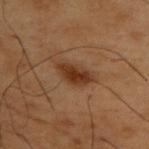Assessment: Imaged during a routine full-body skin examination; the lesion was not biopsied and no histopathology is available. Image and clinical context: A roughly 15 mm field-of-view crop from a total-body skin photograph. The lesion is on the upper back. The total-body-photography lesion software estimated a footprint of about 8 mm² and a shape-asymmetry score of about 0.25 (0 = symmetric). The software also gave border irregularity of about 3 on a 0–10 scale and internal color variation of about 3.5 on a 0–10 scale. This is a cross-polarized tile. A male subject, aged 53 to 57.